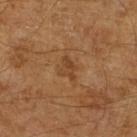workup=total-body-photography surveillance lesion; no biopsy | acquisition=~15 mm crop, total-body skin-cancer survey | patient=male, in their mid-60s | automated metrics=a classifier nevus-likeness of about 0/100.